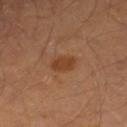No biopsy was performed on this lesion — it was imaged during a full skin examination and was not determined to be concerning. Captured under cross-polarized illumination. Cropped from a whole-body photographic skin survey; the tile spans about 15 mm. About 3 mm across. A male patient, aged approximately 40. The lesion is on the leg.